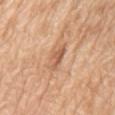| field | value |
|---|---|
| follow-up | total-body-photography surveillance lesion; no biopsy |
| anatomic site | the chest |
| patient | male, roughly 80 years of age |
| imaging modality | ~15 mm crop, total-body skin-cancer survey |
| diameter | about 4 mm |